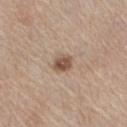Clinical impression:
Captured during whole-body skin photography for melanoma surveillance; the lesion was not biopsied.
Acquisition and patient details:
A lesion tile, about 15 mm wide, cut from a 3D total-body photograph. The lesion is on the left leg. Captured under white-light illumination. The recorded lesion diameter is about 2.5 mm. A female subject, aged 63 to 67.